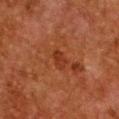Recorded during total-body skin imaging; not selected for excision or biopsy.
The subject is a female aged around 50.
A 15 mm close-up tile from a total-body photography series done for melanoma screening.
From the chest.